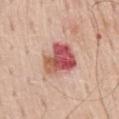  biopsy_status: not biopsied; imaged during a skin examination
  patient:
    sex: male
    age_approx: 60
  image:
    source: total-body photography crop
    field_of_view_mm: 15
  automated_metrics:
    area_mm2_approx: 14.0
    shape_asymmetry: 0.25
    cielab_L: 55
    cielab_a: 30
    cielab_b: 27
    vs_skin_darker_L: 16.0
    vs_skin_contrast_norm: 10.5
    lesion_detection_confidence_0_100: 100
  lighting: white-light
  lesion_size:
    long_diameter_mm_approx: 4.5
  site: mid back